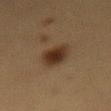| feature | finding |
|---|---|
| follow-up | imaged on a skin check; not biopsied |
| tile lighting | cross-polarized illumination |
| anatomic site | the lower back |
| patient | female, in their mid- to late 50s |
| diameter | about 4 mm |
| automated lesion analysis | roughly 10 lightness units darker than nearby skin; an automated nevus-likeness rating near 100 out of 100 and lesion-presence confidence of about 100/100 |
| imaging modality | total-body-photography crop, ~15 mm field of view |